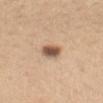subject: female, roughly 65 years of age
automated metrics: a mean CIELAB color near L≈56 a*≈18 b*≈30, a lesion–skin lightness drop of about 16, and a lesion-to-skin contrast of about 10.5 (normalized; higher = more distinct); a border-irregularity rating of about 2/10, a within-lesion color-variation index near 6/10, and a peripheral color-asymmetry measure near 2; a lesion-detection confidence of about 100/100
location: the mid back
imaging modality: ~15 mm tile from a whole-body skin photo
size: ~3 mm (longest diameter)
illumination: white-light